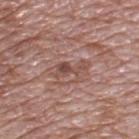The lesion was photographed on a routine skin check and not biopsied; there is no pathology result.
A male patient, approximately 70 years of age.
Captured under white-light illumination.
A roughly 15 mm field-of-view crop from a total-body skin photograph.
Located on the back.
Measured at roughly 3.5 mm in maximum diameter.
An algorithmic analysis of the crop reported a mean CIELAB color near L≈47 a*≈22 b*≈24, about 9 CIELAB-L* units darker than the surrounding skin, and a normalized border contrast of about 7. The analysis additionally found peripheral color asymmetry of about 0.5. The software also gave an automated nevus-likeness rating near 0 out of 100 and lesion-presence confidence of about 100/100.